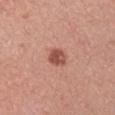Q: Was a biopsy performed?
A: total-body-photography surveillance lesion; no biopsy
Q: What did automated image analysis measure?
A: a footprint of about 4.5 mm², a shape eccentricity near 0.45, and two-axis asymmetry of about 0.2; a normalized border contrast of about 8.5; an automated nevus-likeness rating near 85 out of 100
Q: What is the anatomic site?
A: the left upper arm
Q: Who is the patient?
A: male, approximately 40 years of age
Q: What lighting was used for the tile?
A: white-light illumination
Q: What is the lesion's diameter?
A: ≈2.5 mm
Q: How was this image acquired?
A: 15 mm crop, total-body photography